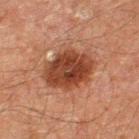A 15 mm close-up tile from a total-body photography series done for melanoma screening. A male subject, about 60 years old. On the left thigh.